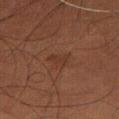The lesion was photographed on a routine skin check and not biopsied; there is no pathology result. A roughly 15 mm field-of-view crop from a total-body skin photograph. A male patient approximately 55 years of age. This is a cross-polarized tile. On the abdomen.